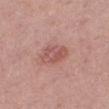follow-up: imaged on a skin check; not biopsied | automated lesion analysis: an average lesion color of about L≈54 a*≈25 b*≈24 (CIELAB) and roughly 9 lightness units darker than nearby skin; a border-irregularity index near 2.5/10, a color-variation rating of about 4.5/10, and a peripheral color-asymmetry measure near 1.5 | subject: female, approximately 40 years of age | body site: the right thigh | tile lighting: white-light illumination | size: about 3.5 mm | image: ~15 mm tile from a whole-body skin photo.